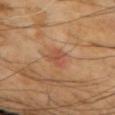Part of a total-body skin-imaging series; this lesion was reviewed on a skin check and was not flagged for biopsy. The tile uses cross-polarized illumination. A 15 mm close-up tile from a total-body photography series done for melanoma screening. The patient is a male approximately 70 years of age. On the left forearm.